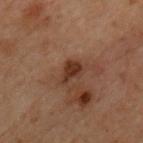Recorded during total-body skin imaging; not selected for excision or biopsy.
A male subject aged 68–72.
Automated tile analysis of the lesion measured an average lesion color of about L≈25 a*≈16 b*≈22 (CIELAB), about 8 CIELAB-L* units darker than the surrounding skin, and a normalized lesion–skin contrast near 9. And it measured a border-irregularity rating of about 3.5/10, a within-lesion color-variation index near 2/10, and a peripheral color-asymmetry measure near 0.5.
Longest diameter approximately 3 mm.
A roughly 15 mm field-of-view crop from a total-body skin photograph.
Located on the chest.